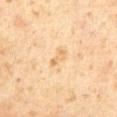Impression: Recorded during total-body skin imaging; not selected for excision or biopsy. Clinical summary: A region of skin cropped from a whole-body photographic capture, roughly 15 mm wide. A male subject, aged 58–62. Located on the front of the torso.